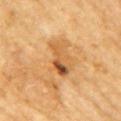Impression:
The lesion was photographed on a routine skin check and not biopsied; there is no pathology result.
Acquisition and patient details:
Imaged with cross-polarized lighting. Cropped from a whole-body photographic skin survey; the tile spans about 15 mm. About 4 mm across. Automated image analysis of the tile measured an average lesion color of about L≈55 a*≈23 b*≈42 (CIELAB), about 11 CIELAB-L* units darker than the surrounding skin, and a normalized border contrast of about 7.5. The analysis additionally found border irregularity of about 3.5 on a 0–10 scale, internal color variation of about 10 on a 0–10 scale, and radial color variation of about 4.5. A female patient aged around 65. The lesion is on the right upper arm.